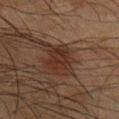<tbp_lesion>
<lesion_size>
  <long_diameter_mm_approx>4.0</long_diameter_mm_approx>
</lesion_size>
<lighting>cross-polarized</lighting>
<patient>
  <sex>male</sex>
  <age_approx>65</age_approx>
</patient>
<image>
  <source>total-body photography crop</source>
  <field_of_view_mm>15</field_of_view_mm>
</image>
<site>mid back</site>
</tbp_lesion>